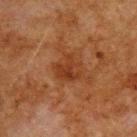This lesion was catalogued during total-body skin photography and was not selected for biopsy.
Imaged with cross-polarized lighting.
The lesion is on the upper back.
The subject is a male aged around 80.
Cropped from a whole-body photographic skin survey; the tile spans about 15 mm.